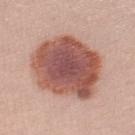Part of a total-body skin-imaging series; this lesion was reviewed on a skin check and was not flagged for biopsy. A female subject approximately 25 years of age. The recorded lesion diameter is about 8 mm. A lesion tile, about 15 mm wide, cut from a 3D total-body photograph. The total-body-photography lesion software estimated a lesion-to-skin contrast of about 11 (normalized; higher = more distinct). The analysis additionally found a within-lesion color-variation index near 6/10. Imaged with white-light lighting. The lesion is on the left lower leg.